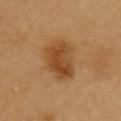Assessment: This lesion was catalogued during total-body skin photography and was not selected for biopsy. Clinical summary: The subject is a male aged 58–62. Approximately 5 mm at its widest. An algorithmic analysis of the crop reported an average lesion color of about L≈45 a*≈22 b*≈38 (CIELAB) and about 10 CIELAB-L* units darker than the surrounding skin. And it measured an automated nevus-likeness rating near 100 out of 100 and lesion-presence confidence of about 100/100. Cropped from a whole-body photographic skin survey; the tile spans about 15 mm. Captured under cross-polarized illumination. From the chest.